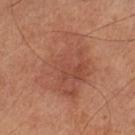biopsy_status: not biopsied; imaged during a skin examination
automated_metrics:
  cielab_L: 47
  cielab_a: 24
  cielab_b: 29
  vs_skin_contrast_norm: 5.0
image:
  source: total-body photography crop
  field_of_view_mm: 15
lighting: cross-polarized
site: left lower leg
patient:
  sex: male
  age_approx: 65
lesion_size:
  long_diameter_mm_approx: 7.5